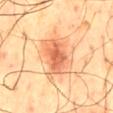This lesion was catalogued during total-body skin photography and was not selected for biopsy. The patient is a male roughly 60 years of age. The lesion's longest dimension is about 6.5 mm. On the mid back. A region of skin cropped from a whole-body photographic capture, roughly 15 mm wide.